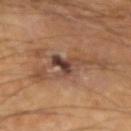Case summary:
• follow-up: no biopsy performed (imaged during a skin exam)